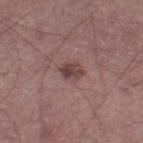| feature | finding |
|---|---|
| biopsy status | imaged on a skin check; not biopsied |
| subject | male, in their 60s |
| acquisition | ~15 mm tile from a whole-body skin photo |
| lighting | white-light |
| size | ~3 mm (longest diameter) |
| body site | the left thigh |